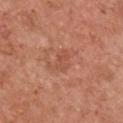The lesion was tiled from a total-body skin photograph and was not biopsied. The recorded lesion diameter is about 3.5 mm. A 15 mm close-up extracted from a 3D total-body photography capture. Captured under white-light illumination. The lesion is located on the chest. An algorithmic analysis of the crop reported a footprint of about 4.5 mm², an outline eccentricity of about 0.75 (0 = round, 1 = elongated), and two-axis asymmetry of about 0.6. The software also gave a border-irregularity index near 6.5/10 and peripheral color asymmetry of about 0.5. The subject is a female approximately 50 years of age.